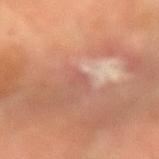biopsy_status: not biopsied; imaged during a skin examination
image:
  source: total-body photography crop
  field_of_view_mm: 15
lesion_size:
  long_diameter_mm_approx: 1.5
lighting: cross-polarized
patient:
  sex: male
automated_metrics:
  cielab_L: 53
  cielab_a: 25
  cielab_b: 28
  vs_skin_darker_L: 6.0
  vs_skin_contrast_norm: 4.5
  border_irregularity_0_10: 4.0
  color_variation_0_10: 0.0
  lesion_detection_confidence_0_100: 10
site: right forearm